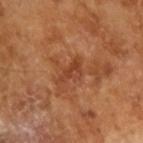| key | value |
|---|---|
| workup | no biopsy performed (imaged during a skin exam) |
| subject | male, aged approximately 65 |
| imaging modality | ~15 mm crop, total-body skin-cancer survey |
| automated metrics | lesion-presence confidence of about 100/100 |
| lighting | cross-polarized illumination |